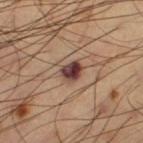workup: catalogued during a skin exam; not biopsied
image source: ~15 mm tile from a whole-body skin photo
subject: male, approximately 60 years of age
location: the leg
tile lighting: cross-polarized illumination
size: ~3 mm (longest diameter)